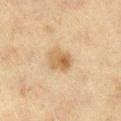<tbp_lesion>
<biopsy_status>not biopsied; imaged during a skin examination</biopsy_status>
<patient>
  <sex>female</sex>
  <age_approx>40</age_approx>
</patient>
<image>
  <source>total-body photography crop</source>
  <field_of_view_mm>15</field_of_view_mm>
</image>
<automated_metrics>
  <eccentricity>0.75</eccentricity>
  <cielab_L>54</cielab_L>
  <cielab_a>15</cielab_a>
  <cielab_b>34</cielab_b>
  <vs_skin_contrast_norm>7.5</vs_skin_contrast_norm>
  <nevus_likeness_0_100>65</nevus_likeness_0_100>
  <lesion_detection_confidence_0_100>100</lesion_detection_confidence_0_100>
</automated_metrics>
<lesion_size>
  <long_diameter_mm_approx>3.5</long_diameter_mm_approx>
</lesion_size>
<site>right lower leg</site>
</tbp_lesion>